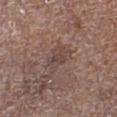Q: Was this lesion biopsied?
A: total-body-photography surveillance lesion; no biopsy
Q: What is the anatomic site?
A: the left lower leg
Q: How was this image acquired?
A: ~15 mm crop, total-body skin-cancer survey
Q: Who is the patient?
A: male, roughly 70 years of age